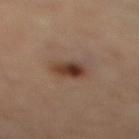Notes:
* follow-up: imaged on a skin check; not biopsied
* tile lighting: cross-polarized
* size: about 3 mm
* TBP lesion metrics: a lesion color around L≈39 a*≈19 b*≈27 in CIELAB, roughly 13 lightness units darker than nearby skin, and a normalized lesion–skin contrast near 10.5
* image source: 15 mm crop, total-body photography
* subject: female, roughly 70 years of age
* location: the right thigh